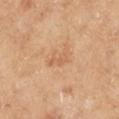This lesion was catalogued during total-body skin photography and was not selected for biopsy.
The tile uses cross-polarized illumination.
The subject is a female in their 60s.
A roughly 15 mm field-of-view crop from a total-body skin photograph.
The lesion is located on the right lower leg.
Automated tile analysis of the lesion measured a border-irregularity rating of about 4.5/10, a color-variation rating of about 0.5/10, and peripheral color asymmetry of about 0. The software also gave a classifier nevus-likeness of about 0/100 and a lesion-detection confidence of about 100/100.